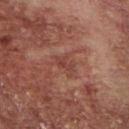Case summary:
- follow-up · imaged on a skin check; not biopsied
- acquisition · ~15 mm tile from a whole-body skin photo
- lesion size · ≈2.5 mm
- anatomic site · the chest
- subject · male, about 55 years old